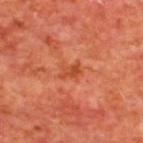notes: total-body-photography surveillance lesion; no biopsy
lesion size: ≈2.5 mm
patient: male, in their mid- to late 60s
tile lighting: cross-polarized
anatomic site: the upper back
image source: 15 mm crop, total-body photography